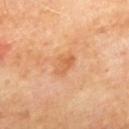The lesion is on the mid back. The patient is a male roughly 70 years of age. A 15 mm close-up tile from a total-body photography series done for melanoma screening.